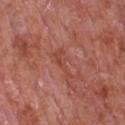Impression: The lesion was photographed on a routine skin check and not biopsied; there is no pathology result. Acquisition and patient details: A male patient about 65 years old. A lesion tile, about 15 mm wide, cut from a 3D total-body photograph. The lesion's longest dimension is about 4 mm. From the chest. Imaged with white-light lighting.